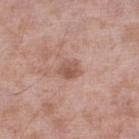biopsy status — no biopsy performed (imaged during a skin exam)
image source — total-body-photography crop, ~15 mm field of view
illumination — white-light illumination
patient — male, about 55 years old
anatomic site — the left lower leg
TBP lesion metrics — a footprint of about 4 mm², an outline eccentricity of about 0.55 (0 = round, 1 = elongated), and a shape-asymmetry score of about 0.2 (0 = symmetric); a lesion–skin lightness drop of about 10 and a lesion-to-skin contrast of about 7 (normalized; higher = more distinct); border irregularity of about 2 on a 0–10 scale, internal color variation of about 3 on a 0–10 scale, and radial color variation of about 1